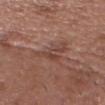Assessment:
Recorded during total-body skin imaging; not selected for excision or biopsy.
Acquisition and patient details:
A male patient aged around 55. The lesion's longest dimension is about 4 mm. Captured under white-light illumination. A region of skin cropped from a whole-body photographic capture, roughly 15 mm wide. The lesion is on the head or neck.